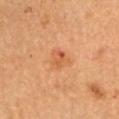This lesion was catalogued during total-body skin photography and was not selected for biopsy. The lesion-visualizer software estimated a lesion area of about 5 mm², a shape eccentricity near 0.55, and a symmetry-axis asymmetry near 0.3. The analysis additionally found a border-irregularity index near 3/10 and radial color variation of about 1.5. A male patient, approximately 65 years of age. Measured at roughly 3 mm in maximum diameter. The lesion is located on the left upper arm. Cropped from a total-body skin-imaging series; the visible field is about 15 mm.